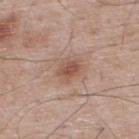workup: catalogued during a skin exam; not biopsied
illumination: white-light illumination
subject: male, aged 63 to 67
lesion size: about 3 mm
imaging modality: ~15 mm tile from a whole-body skin photo
TBP lesion metrics: a lesion area of about 6.5 mm², an outline eccentricity of about 0.55 (0 = round, 1 = elongated), and a shape-asymmetry score of about 0.25 (0 = symmetric); a nevus-likeness score of about 55/100
body site: the upper back